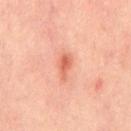Part of a total-body skin-imaging series; this lesion was reviewed on a skin check and was not flagged for biopsy.
A male patient aged 43 to 47.
Longest diameter approximately 3 mm.
A lesion tile, about 15 mm wide, cut from a 3D total-body photograph.
The lesion is on the chest.